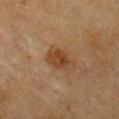biopsy status: catalogued during a skin exam; not biopsied
image source: 15 mm crop, total-body photography
site: the chest
patient: female, in their mid-50s
automated metrics: a lesion area of about 6.5 mm² and an outline eccentricity of about 0.75 (0 = round, 1 = elongated); lesion-presence confidence of about 100/100
lesion size: ≈3.5 mm
illumination: cross-polarized illumination